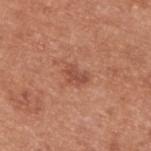Clinical impression: The lesion was photographed on a routine skin check and not biopsied; there is no pathology result. Acquisition and patient details: An algorithmic analysis of the crop reported an outline eccentricity of about 0.75 (0 = round, 1 = elongated) and a shape-asymmetry score of about 0.3 (0 = symmetric). The analysis additionally found a lesion-to-skin contrast of about 6 (normalized; higher = more distinct). It also reported a border-irregularity rating of about 3/10, a within-lesion color-variation index near 2/10, and peripheral color asymmetry of about 0.5. Cropped from a total-body skin-imaging series; the visible field is about 15 mm. This is a white-light tile. A male subject in their mid-50s. Located on the upper back.